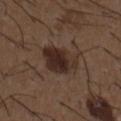Captured during whole-body skin photography for melanoma surveillance; the lesion was not biopsied. Located on the front of the torso. Longest diameter approximately 4.5 mm. Cropped from a whole-body photographic skin survey; the tile spans about 15 mm. The subject is a male in their 50s.